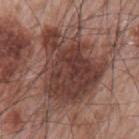Clinical impression: The lesion was tiled from a total-body skin photograph and was not biopsied. Image and clinical context: About 8 mm across. This image is a 15 mm lesion crop taken from a total-body photograph. The tile uses white-light illumination. On the left upper arm. An algorithmic analysis of the crop reported a footprint of about 37 mm², an outline eccentricity of about 0.55 (0 = round, 1 = elongated), and a shape-asymmetry score of about 0.4 (0 = symmetric). The analysis additionally found an average lesion color of about L≈39 a*≈20 b*≈23 (CIELAB), a lesion–skin lightness drop of about 12, and a normalized lesion–skin contrast near 10. The software also gave an automated nevus-likeness rating near 75 out of 100 and a lesion-detection confidence of about 100/100. The patient is a male aged approximately 65.